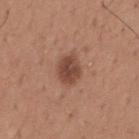Assessment: This lesion was catalogued during total-body skin photography and was not selected for biopsy. Image and clinical context: The lesion is located on the mid back. This image is a 15 mm lesion crop taken from a total-body photograph. A male subject, roughly 65 years of age. The tile uses white-light illumination.